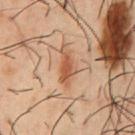{
  "biopsy_status": "not biopsied; imaged during a skin examination",
  "automated_metrics": {
    "area_mm2_approx": 6.5,
    "shape_asymmetry": 0.3,
    "vs_skin_darker_L": 10.0,
    "vs_skin_contrast_norm": 7.5,
    "border_irregularity_0_10": 4.0,
    "color_variation_0_10": 3.0,
    "peripheral_color_asymmetry": 1.0
  },
  "patient": {
    "sex": "male",
    "age_approx": 55
  },
  "lesion_size": {
    "long_diameter_mm_approx": 4.5
  },
  "image": {
    "source": "total-body photography crop",
    "field_of_view_mm": 15
  },
  "site": "chest"
}